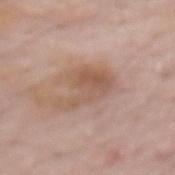{"biopsy_status": "not biopsied; imaged during a skin examination", "automated_metrics": {"cielab_L": 56, "cielab_a": 18, "cielab_b": 28, "vs_skin_darker_L": 8.0, "vs_skin_contrast_norm": 6.0, "nevus_likeness_0_100": 10, "lesion_detection_confidence_0_100": 100}, "site": "mid back", "patient": {"sex": "male", "age_approx": 70}, "image": {"source": "total-body photography crop", "field_of_view_mm": 15}, "lesion_size": {"long_diameter_mm_approx": 5.5}}